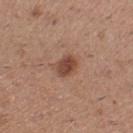Q: Is there a histopathology result?
A: imaged on a skin check; not biopsied
Q: Patient demographics?
A: female, about 30 years old
Q: What is the anatomic site?
A: the right lower leg
Q: What is the lesion's diameter?
A: ~2.5 mm (longest diameter)
Q: What is the imaging modality?
A: ~15 mm crop, total-body skin-cancer survey
Q: Automated lesion metrics?
A: a lesion area of about 5 mm², an eccentricity of roughly 0.65, and a symmetry-axis asymmetry near 0.15; a border-irregularity index near 1.5/10; a nevus-likeness score of about 95/100 and a detector confidence of about 100 out of 100 that the crop contains a lesion